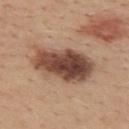image source: total-body-photography crop, ~15 mm field of view | illumination: white-light | automated lesion analysis: a footprint of about 23 mm², an outline eccentricity of about 0.85 (0 = round, 1 = elongated), and a shape-asymmetry score of about 0.2 (0 = symmetric); an automated nevus-likeness rating near 90 out of 100 | site: the upper back | size: about 7.5 mm | subject: female, aged around 45.